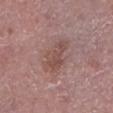<tbp_lesion>
  <patient>
    <sex>male</sex>
    <age_approx>75</age_approx>
  </patient>
  <automated_metrics>
    <eccentricity>0.85</eccentricity>
    <shape_asymmetry>0.4</shape_asymmetry>
  </automated_metrics>
  <lesion_size>
    <long_diameter_mm_approx>4.5</long_diameter_mm_approx>
  </lesion_size>
  <lighting>white-light</lighting>
  <site>left lower leg</site>
  <image>
    <source>total-body photography crop</source>
    <field_of_view_mm>15</field_of_view_mm>
  </image>
</tbp_lesion>